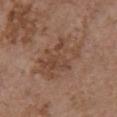<tbp_lesion>
  <biopsy_status>not biopsied; imaged during a skin examination</biopsy_status>
  <image>
    <source>total-body photography crop</source>
    <field_of_view_mm>15</field_of_view_mm>
  </image>
  <lesion_size>
    <long_diameter_mm_approx>7.0</long_diameter_mm_approx>
  </lesion_size>
  <lighting>white-light</lighting>
  <patient>
    <sex>female</sex>
    <age_approx>65</age_approx>
  </patient>
  <site>chest</site>
</tbp_lesion>